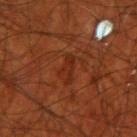Q: Was this lesion biopsied?
A: catalogued during a skin exam; not biopsied
Q: Who is the patient?
A: male, in their 70s
Q: Where on the body is the lesion?
A: the right upper arm
Q: What is the imaging modality?
A: total-body-photography crop, ~15 mm field of view
Q: Automated lesion metrics?
A: an area of roughly 4 mm² and a shape-asymmetry score of about 0.35 (0 = symmetric); a color-variation rating of about 1/10 and a peripheral color-asymmetry measure near 0
Q: Illumination type?
A: cross-polarized illumination
Q: Lesion size?
A: about 3.5 mm